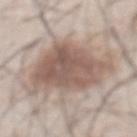patient = male, aged 53 to 57 | anatomic site = the abdomen | tile lighting = white-light | imaging modality = ~15 mm crop, total-body skin-cancer survey | lesion diameter = about 7.5 mm.